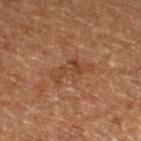notes: total-body-photography surveillance lesion; no biopsy
tile lighting: cross-polarized
image source: ~15 mm tile from a whole-body skin photo
lesion size: about 5 mm
subject: male, aged 73 to 77
location: the left lower leg